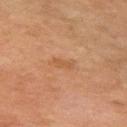| feature | finding |
|---|---|
| biopsy status | imaged on a skin check; not biopsied |
| lighting | cross-polarized illumination |
| location | the right upper arm |
| subject | female, aged 58–62 |
| automated lesion analysis | an area of roughly 3.5 mm² and a symmetry-axis asymmetry near 0.35; a border-irregularity index near 3.5/10 and internal color variation of about 1 on a 0–10 scale; a nevus-likeness score of about 0/100 and a lesion-detection confidence of about 100/100 |
| lesion size | about 3 mm |
| acquisition | total-body-photography crop, ~15 mm field of view |